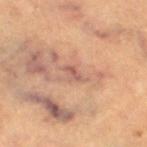Part of a total-body skin-imaging series; this lesion was reviewed on a skin check and was not flagged for biopsy. The recorded lesion diameter is about 2.5 mm. An algorithmic analysis of the crop reported a lesion color around L≈53 a*≈22 b*≈26 in CIELAB, roughly 9 lightness units darker than nearby skin, and a normalized lesion–skin contrast near 6.5. And it measured a border-irregularity rating of about 3.5/10, internal color variation of about 0 on a 0–10 scale, and a peripheral color-asymmetry measure near 0. And it measured a classifier nevus-likeness of about 0/100 and a detector confidence of about 70 out of 100 that the crop contains a lesion. A female subject, in their 60s. Captured under cross-polarized illumination. A lesion tile, about 15 mm wide, cut from a 3D total-body photograph. The lesion is located on the left thigh.